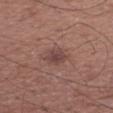Case summary:
• biopsy status · total-body-photography surveillance lesion; no biopsy
• TBP lesion metrics · a border-irregularity index near 2/10, a within-lesion color-variation index near 2/10, and a peripheral color-asymmetry measure near 0.5
• subject · male, aged approximately 50
• anatomic site · the right forearm
• image source · 15 mm crop, total-body photography
• illumination · white-light